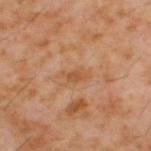Captured during whole-body skin photography for melanoma surveillance; the lesion was not biopsied. From the upper back. A 15 mm close-up extracted from a 3D total-body photography capture. A male patient, aged 58–62. The lesion's longest dimension is about 3 mm.